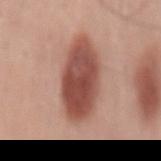Imaged during a routine full-body skin examination; the lesion was not biopsied and no histopathology is available.
Automated tile analysis of the lesion measured an average lesion color of about L≈50 a*≈25 b*≈27 (CIELAB), a lesion–skin lightness drop of about 16, and a normalized lesion–skin contrast near 10.5. The analysis additionally found lesion-presence confidence of about 100/100.
Longest diameter approximately 8.5 mm.
Located on the mid back.
A female subject, aged around 40.
Cropped from a whole-body photographic skin survey; the tile spans about 15 mm.
Imaged with white-light lighting.